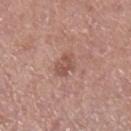Captured during whole-body skin photography for melanoma surveillance; the lesion was not biopsied. The subject is a male aged around 55. The tile uses white-light illumination. From the left lower leg. A close-up tile cropped from a whole-body skin photograph, about 15 mm across. Longest diameter approximately 2.5 mm.